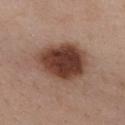Q: Was a biopsy performed?
A: catalogued during a skin exam; not biopsied
Q: What is the imaging modality?
A: 15 mm crop, total-body photography
Q: Where on the body is the lesion?
A: the chest
Q: How large is the lesion?
A: about 7 mm
Q: Who is the patient?
A: female, about 55 years old